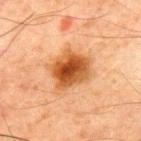Assessment: Part of a total-body skin-imaging series; this lesion was reviewed on a skin check and was not flagged for biopsy. Acquisition and patient details: The lesion is located on the chest. A 15 mm close-up tile from a total-body photography series done for melanoma screening. A male subject, in their 70s.